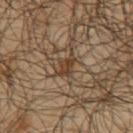| field | value |
|---|---|
| workup | catalogued during a skin exam; not biopsied |
| patient | male, approximately 65 years of age |
| image source | ~15 mm crop, total-body skin-cancer survey |
| diameter | ≈3 mm |
| site | the upper back |